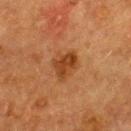notes: total-body-photography surveillance lesion; no biopsy
subject: female, aged around 55
size: ≈3.5 mm
illumination: cross-polarized illumination
imaging modality: total-body-photography crop, ~15 mm field of view
site: the upper back